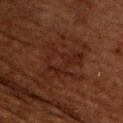Assessment:
The lesion was photographed on a routine skin check and not biopsied; there is no pathology result.